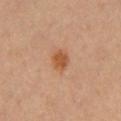Case summary:
• workup · catalogued during a skin exam; not biopsied
• image · total-body-photography crop, ~15 mm field of view
• TBP lesion metrics · an area of roughly 5 mm²; a mean CIELAB color near L≈50 a*≈22 b*≈36, a lesion–skin lightness drop of about 9, and a normalized border contrast of about 8; border irregularity of about 2 on a 0–10 scale; an automated nevus-likeness rating near 95 out of 100 and a detector confidence of about 100 out of 100 that the crop contains a lesion
• lighting · cross-polarized
• lesion size · about 3 mm
• anatomic site · the chest
• patient · female, roughly 30 years of age